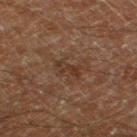A roughly 15 mm field-of-view crop from a total-body skin photograph.
A male patient approximately 60 years of age.
This is a cross-polarized tile.
Automated tile analysis of the lesion measured a border-irregularity index near 5.5/10, a within-lesion color-variation index near 0/10, and radial color variation of about 0.
On the left thigh.
The lesion's longest dimension is about 3 mm.